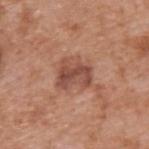{"biopsy_status": "not biopsied; imaged during a skin examination", "site": "upper back", "image": {"source": "total-body photography crop", "field_of_view_mm": 15}, "automated_metrics": {"cielab_L": 49, "cielab_a": 24, "cielab_b": 28, "vs_skin_darker_L": 10.0, "vs_skin_contrast_norm": 7.5, "border_irregularity_0_10": 3.5, "color_variation_0_10": 5.0, "nevus_likeness_0_100": 20}, "lighting": "white-light", "patient": {"sex": "male", "age_approx": 65}}